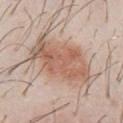This lesion was catalogued during total-body skin photography and was not selected for biopsy. The recorded lesion diameter is about 9 mm. The patient is a male aged 28 to 32. On the front of the torso. Automated tile analysis of the lesion measured a shape eccentricity near 0.85. A close-up tile cropped from a whole-body skin photograph, about 15 mm across.